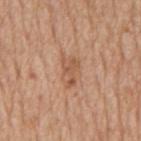Case summary:
– follow-up · no biopsy performed (imaged during a skin exam)
– image-analysis metrics · an area of roughly 6 mm² and a shape-asymmetry score of about 0.35 (0 = symmetric); a lesion color around L≈56 a*≈22 b*≈33 in CIELAB, roughly 8 lightness units darker than nearby skin, and a lesion-to-skin contrast of about 6 (normalized; higher = more distinct); a border-irregularity rating of about 3.5/10; a classifier nevus-likeness of about 0/100 and a detector confidence of about 100 out of 100 that the crop contains a lesion
– lighting · white-light illumination
– subject · male, aged around 65
– image · ~15 mm tile from a whole-body skin photo
– location · the mid back